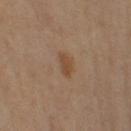biopsy_status: not biopsied; imaged during a skin examination
image:
  source: total-body photography crop
  field_of_view_mm: 15
patient:
  sex: female
  age_approx: 65
lighting: cross-polarized
automated_metrics:
  cielab_L: 44
  cielab_a: 17
  cielab_b: 30
  vs_skin_darker_L: 7.0
  vs_skin_contrast_norm: 7.0
  nevus_likeness_0_100: 65
  lesion_detection_confidence_0_100: 100
lesion_size:
  long_diameter_mm_approx: 3.0
site: arm